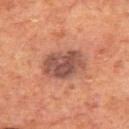Image and clinical context: The tile uses cross-polarized illumination. An algorithmic analysis of the crop reported an eccentricity of roughly 0.8 and a symmetry-axis asymmetry near 0.15. And it measured an average lesion color of about L≈47 a*≈22 b*≈26 (CIELAB), roughly 12 lightness units darker than nearby skin, and a normalized border contrast of about 9.5. And it measured a classifier nevus-likeness of about 50/100. From the back. A male subject in their 70s. Approximately 5.5 mm at its widest. A 15 mm crop from a total-body photograph taken for skin-cancer surveillance. Diagnosis: On biopsy, histopathology showed a lichen planus-like keratosis.